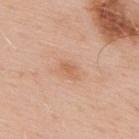| key | value |
|---|---|
| workup | no biopsy performed (imaged during a skin exam) |
| lesion size | about 2.5 mm |
| image-analysis metrics | a mean CIELAB color near L≈62 a*≈22 b*≈35, a lesion–skin lightness drop of about 7, and a lesion-to-skin contrast of about 5.5 (normalized; higher = more distinct); a border-irregularity rating of about 2/10 and a within-lesion color-variation index near 2.5/10; a nevus-likeness score of about 0/100 |
| patient | male, in their mid- to late 50s |
| site | the upper back |
| image source | 15 mm crop, total-body photography |
| lighting | white-light |